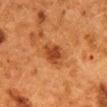workup — imaged on a skin check; not biopsied
image source — 15 mm crop, total-body photography
patient — female, approximately 50 years of age
location — the mid back